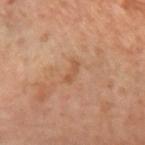Assessment: This lesion was catalogued during total-body skin photography and was not selected for biopsy. Background: The lesion is located on the left forearm. About 2.5 mm across. The tile uses cross-polarized illumination. A female patient, roughly 65 years of age. A lesion tile, about 15 mm wide, cut from a 3D total-body photograph. An algorithmic analysis of the crop reported a shape eccentricity near 0.95 and two-axis asymmetry of about 0.45. The analysis additionally found a lesion color around L≈52 a*≈22 b*≈34 in CIELAB, about 7 CIELAB-L* units darker than the surrounding skin, and a normalized border contrast of about 5.5. The software also gave a border-irregularity index near 5/10 and radial color variation of about 0.